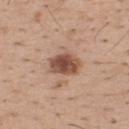{
  "biopsy_status": "not biopsied; imaged during a skin examination",
  "site": "mid back",
  "lesion_size": {
    "long_diameter_mm_approx": 4.0
  },
  "patient": {
    "sex": "male",
    "age_approx": 40
  },
  "image": {
    "source": "total-body photography crop",
    "field_of_view_mm": 15
  }
}